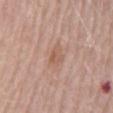Impression:
Recorded during total-body skin imaging; not selected for excision or biopsy.
Clinical summary:
A close-up tile cropped from a whole-body skin photograph, about 15 mm across. From the mid back. Longest diameter approximately 2.5 mm. A female patient, in their mid-60s. This is a white-light tile. The total-body-photography lesion software estimated a lesion area of about 4.5 mm², a shape eccentricity near 0.7, and two-axis asymmetry of about 0.25. The analysis additionally found border irregularity of about 2 on a 0–10 scale, internal color variation of about 3 on a 0–10 scale, and a peripheral color-asymmetry measure near 1.